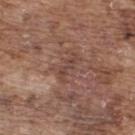Clinical impression: Part of a total-body skin-imaging series; this lesion was reviewed on a skin check and was not flagged for biopsy. Image and clinical context: Cropped from a total-body skin-imaging series; the visible field is about 15 mm. Longest diameter approximately 3.5 mm. From the upper back. The subject is a male in their mid- to late 70s.